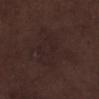Q: How was this image acquired?
A: ~15 mm tile from a whole-body skin photo
Q: What is the anatomic site?
A: the left lower leg
Q: What is the lesion's diameter?
A: ~6 mm (longest diameter)
Q: What lighting was used for the tile?
A: white-light illumination
Q: Automated lesion metrics?
A: a mean CIELAB color near L≈22 a*≈14 b*≈14 and about 3 CIELAB-L* units darker than the surrounding skin; a border-irregularity rating of about 5.5/10, internal color variation of about 2 on a 0–10 scale, and a peripheral color-asymmetry measure near 0.5; a classifier nevus-likeness of about 0/100
Q: What are the patient's age and sex?
A: male, roughly 70 years of age